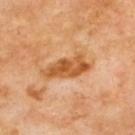Imaged during a routine full-body skin examination; the lesion was not biopsied and no histopathology is available.
A 15 mm close-up tile from a total-body photography series done for melanoma screening.
On the back.
The recorded lesion diameter is about 5.5 mm.
A male subject approximately 70 years of age.
The total-body-photography lesion software estimated a lesion area of about 11 mm². The software also gave a border-irregularity rating of about 4/10 and a color-variation rating of about 4.5/10. And it measured a nevus-likeness score of about 0/100 and a detector confidence of about 100 out of 100 that the crop contains a lesion.